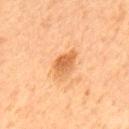Image and clinical context: Cropped from a whole-body photographic skin survey; the tile spans about 15 mm. From the upper back. A male patient in their mid-60s. Measured at roughly 4 mm in maximum diameter. The lesion-visualizer software estimated about 10 CIELAB-L* units darker than the surrounding skin and a normalized lesion–skin contrast near 7.5. It also reported border irregularity of about 2 on a 0–10 scale, a within-lesion color-variation index near 4/10, and radial color variation of about 1.5. It also reported an automated nevus-likeness rating near 75 out of 100 and a detector confidence of about 100 out of 100 that the crop contains a lesion. Captured under cross-polarized illumination.